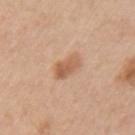On the arm. The recorded lesion diameter is about 3.5 mm. A male patient aged approximately 70. A close-up tile cropped from a whole-body skin photograph, about 15 mm across.